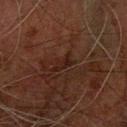Findings:
* notes · imaged on a skin check; not biopsied